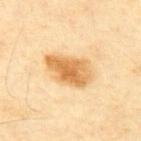No biopsy was performed on this lesion — it was imaged during a full skin examination and was not determined to be concerning. A male subject approximately 60 years of age. A lesion tile, about 15 mm wide, cut from a 3D total-body photograph. Measured at roughly 5.5 mm in maximum diameter. On the mid back. An algorithmic analysis of the crop reported a footprint of about 14 mm², a shape eccentricity near 0.8, and a symmetry-axis asymmetry near 0.25. The analysis additionally found a lesion-detection confidence of about 100/100. This is a cross-polarized tile.